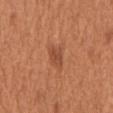The lesion was photographed on a routine skin check and not biopsied; there is no pathology result. From the chest. Captured under white-light illumination. A male patient in their mid-60s. A 15 mm crop from a total-body photograph taken for skin-cancer surveillance. Automated image analysis of the tile measured an average lesion color of about L≈49 a*≈26 b*≈34 (CIELAB) and about 8 CIELAB-L* units darker than the surrounding skin. It also reported border irregularity of about 2.5 on a 0–10 scale, internal color variation of about 2 on a 0–10 scale, and peripheral color asymmetry of about 1.